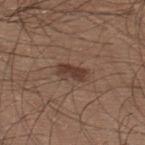notes — imaged on a skin check; not biopsied | size — ≈3.5 mm | site — the back | TBP lesion metrics — a lesion area of about 6 mm²; an average lesion color of about L≈39 a*≈18 b*≈24 (CIELAB) and a lesion-to-skin contrast of about 8 (normalized; higher = more distinct); a classifier nevus-likeness of about 85/100 and a lesion-detection confidence of about 100/100 | subject — male, aged approximately 25 | image — ~15 mm tile from a whole-body skin photo | lighting — white-light.